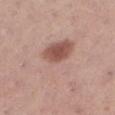Q: Was a biopsy performed?
A: imaged on a skin check; not biopsied